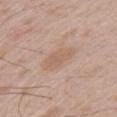Notes:
- notes: total-body-photography surveillance lesion; no biopsy
- imaging modality: 15 mm crop, total-body photography
- site: the arm
- patient: male, about 50 years old